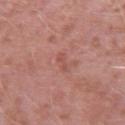Findings:
- notes: imaged on a skin check; not biopsied
- image source: 15 mm crop, total-body photography
- site: the right upper arm
- subject: male, aged around 40